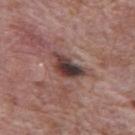The lesion was photographed on a routine skin check and not biopsied; there is no pathology result.
Automated image analysis of the tile measured a lesion color around L≈39 a*≈18 b*≈19 in CIELAB, about 15 CIELAB-L* units darker than the surrounding skin, and a normalized border contrast of about 11.5. It also reported internal color variation of about 10 on a 0–10 scale.
This is a white-light tile.
The lesion is located on the mid back.
Measured at roughly 4.5 mm in maximum diameter.
A male subject, in their 70s.
A 15 mm close-up extracted from a 3D total-body photography capture.